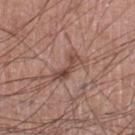The lesion was photographed on a routine skin check and not biopsied; there is no pathology result. On the left lower leg. This image is a 15 mm lesion crop taken from a total-body photograph. The subject is a male roughly 60 years of age.